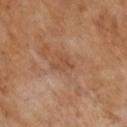Cropped from a total-body skin-imaging series; the visible field is about 15 mm. The subject is a male about 65 years old.